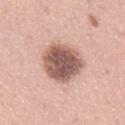image source = total-body-photography crop, ~15 mm field of view | illumination = white-light illumination | body site = the lower back | automated lesion analysis = a lesion area of about 18 mm²; border irregularity of about 1.5 on a 0–10 scale and peripheral color asymmetry of about 1.5 | patient = female, aged around 30 | size = about 5 mm.